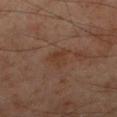Recorded during total-body skin imaging; not selected for excision or biopsy.
The lesion is on the right lower leg.
A 15 mm close-up tile from a total-body photography series done for melanoma screening.
A male subject aged 53 to 57.
The recorded lesion diameter is about 2.5 mm.
Captured under cross-polarized illumination.